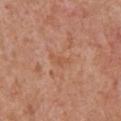Q: Was a biopsy performed?
A: imaged on a skin check; not biopsied
Q: What is the lesion's diameter?
A: ~2.5 mm (longest diameter)
Q: Patient demographics?
A: male, aged approximately 65
Q: What is the anatomic site?
A: the chest
Q: How was this image acquired?
A: ~15 mm tile from a whole-body skin photo
Q: How was the tile lit?
A: white-light